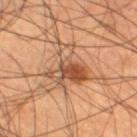The lesion was tiled from a total-body skin photograph and was not biopsied.
On the left thigh.
The lesion's longest dimension is about 6 mm.
A male patient aged 43–47.
Imaged with cross-polarized lighting.
A 15 mm close-up extracted from a 3D total-body photography capture.
Automated image analysis of the tile measured an area of roughly 14 mm². And it measured border irregularity of about 8.5 on a 0–10 scale, internal color variation of about 9.5 on a 0–10 scale, and radial color variation of about 3.